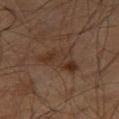About 5 mm across.
A male subject roughly 60 years of age.
A 15 mm crop from a total-body photograph taken for skin-cancer surveillance.
Imaged with cross-polarized lighting.
Automated tile analysis of the lesion measured an area of roughly 11 mm², a shape eccentricity near 0.8, and two-axis asymmetry of about 0.45. The software also gave a nevus-likeness score of about 0/100 and a detector confidence of about 100 out of 100 that the crop contains a lesion.
From the left thigh.